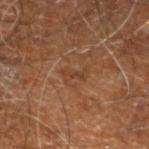<lesion>
<lesion_size>
  <long_diameter_mm_approx>3.0</long_diameter_mm_approx>
</lesion_size>
<image>
  <source>total-body photography crop</source>
  <field_of_view_mm>15</field_of_view_mm>
</image>
<site>right leg</site>
<lighting>cross-polarized</lighting>
<automated_metrics>
  <vs_skin_darker_L>6.0</vs_skin_darker_L>
  <vs_skin_contrast_norm>5.0</vs_skin_contrast_norm>
  <border_irregularity_0_10>6.0</border_irregularity_0_10>
  <color_variation_0_10>0.0</color_variation_0_10>
  <peripheral_color_asymmetry>0.0</peripheral_color_asymmetry>
  <nevus_likeness_0_100>0</nevus_likeness_0_100>
  <lesion_detection_confidence_0_100>65</lesion_detection_confidence_0_100>
</automated_metrics>
<patient>
  <sex>male</sex>
  <age_approx>60</age_approx>
</patient>
</lesion>